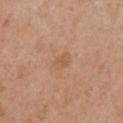  biopsy_status: not biopsied; imaged during a skin examination
  site: chest
  lesion_size:
    long_diameter_mm_approx: 2.5
  patient:
    sex: female
    age_approx: 40
  image:
    source: total-body photography crop
    field_of_view_mm: 15
  lighting: white-light